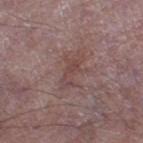No biopsy was performed on this lesion — it was imaged during a full skin examination and was not determined to be concerning.
Captured under white-light illumination.
Cropped from a total-body skin-imaging series; the visible field is about 15 mm.
On the right thigh.
A male subject, roughly 65 years of age.